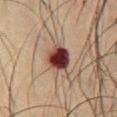Notes:
- notes: no biopsy performed (imaged during a skin exam)
- body site: the front of the torso
- imaging modality: ~15 mm tile from a whole-body skin photo
- patient: male, in their 60s